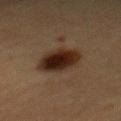<case>
<patient>
  <sex>female</sex>
  <age_approx>55</age_approx>
</patient>
<site>mid back</site>
<image>
  <source>total-body photography crop</source>
  <field_of_view_mm>15</field_of_view_mm>
</image>
<lighting>cross-polarized</lighting>
<lesion_size>
  <long_diameter_mm_approx>5.5</long_diameter_mm_approx>
</lesion_size>
<diagnosis>
  <histopathology>melanocytic nevus</histopathology>
  <malignancy>benign</malignancy>
  <taxonomic_path>Benign, Benign melanocytic proliferations, Nevus</taxonomic_path>
</diagnosis>
</case>